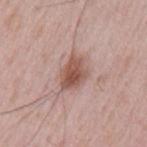follow-up — total-body-photography surveillance lesion; no biopsy
subject — male, in their 50s
automated metrics — an area of roughly 8.5 mm², a shape eccentricity near 0.7, and a shape-asymmetry score of about 0.3 (0 = symmetric); an average lesion color of about L≈53 a*≈21 b*≈25 (CIELAB), a lesion–skin lightness drop of about 12, and a normalized border contrast of about 8.5; a within-lesion color-variation index near 4.5/10 and peripheral color asymmetry of about 1.5
imaging modality — ~15 mm tile from a whole-body skin photo
lighting — white-light illumination
size — about 4 mm
body site — the left upper arm